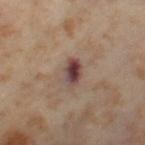image source = total-body-photography crop, ~15 mm field of view
lighting = cross-polarized illumination
body site = the leg
subject = female, aged 53–57
lesion size = ~3 mm (longest diameter)